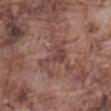Notes:
* follow-up · no biopsy performed (imaged during a skin exam)
* imaging modality · total-body-photography crop, ~15 mm field of view
* subject · male, in their mid-70s
* lesion size · ~5 mm (longest diameter)
* illumination · white-light illumination
* location · the abdomen